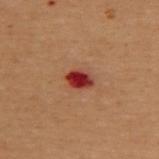biopsy status — catalogued during a skin exam; not biopsied
site — the upper back
illumination — cross-polarized
automated lesion analysis — a mean CIELAB color near L≈31 a*≈32 b*≈27, about 15 CIELAB-L* units darker than the surrounding skin, and a lesion-to-skin contrast of about 13.5 (normalized; higher = more distinct); border irregularity of about 1 on a 0–10 scale, a color-variation rating of about 3.5/10, and radial color variation of about 1; a lesion-detection confidence of about 100/100
lesion diameter — ~3 mm (longest diameter)
subject — female, approximately 50 years of age
image — total-body-photography crop, ~15 mm field of view